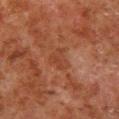Clinical impression: This lesion was catalogued during total-body skin photography and was not selected for biopsy. Clinical summary: The subject is a male aged 78 to 82. Cropped from a total-body skin-imaging series; the visible field is about 15 mm. The tile uses cross-polarized illumination. Located on the right lower leg.